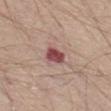The tile uses white-light illumination. The lesion is located on the left thigh. Cropped from a whole-body photographic skin survey; the tile spans about 15 mm. The patient is a male approximately 65 years of age.